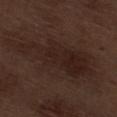Assessment: No biopsy was performed on this lesion — it was imaged during a full skin examination and was not determined to be concerning. Clinical summary: A 15 mm crop from a total-body photograph taken for skin-cancer surveillance. Located on the right thigh. A male subject, roughly 70 years of age.